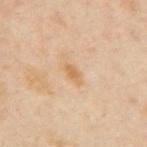Captured during whole-body skin photography for melanoma surveillance; the lesion was not biopsied. This is a cross-polarized tile. The lesion-visualizer software estimated an automated nevus-likeness rating near 0 out of 100 and a lesion-detection confidence of about 100/100. A roughly 15 mm field-of-view crop from a total-body skin photograph. Approximately 2.5 mm at its widest. The lesion is on the left arm. The subject is a female aged 38 to 42.